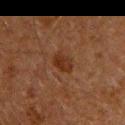  biopsy_status: not biopsied; imaged during a skin examination
  patient:
    sex: male
    age_approx: 60
  image:
    source: total-body photography crop
    field_of_view_mm: 15
  lesion_size:
    long_diameter_mm_approx: 2.5
  site: chest
  lighting: cross-polarized